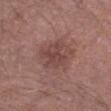biopsy status=total-body-photography surveillance lesion; no biopsy | patient=male, about 70 years old | imaging modality=total-body-photography crop, ~15 mm field of view | illumination=white-light illumination | site=the leg.